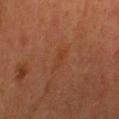Clinical impression:
Imaged during a routine full-body skin examination; the lesion was not biopsied and no histopathology is available.
Context:
A female patient aged 53 to 57. From the front of the torso. Captured under cross-polarized illumination. A 15 mm close-up extracted from a 3D total-body photography capture.